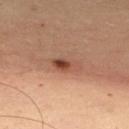| key | value |
|---|---|
| notes | total-body-photography surveillance lesion; no biopsy |
| TBP lesion metrics | a nevus-likeness score of about 90/100 and a detector confidence of about 100 out of 100 that the crop contains a lesion |
| patient | female, about 45 years old |
| site | the front of the torso |
| diameter | ≈4 mm |
| image | total-body-photography crop, ~15 mm field of view |
| tile lighting | cross-polarized |